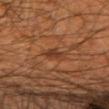biopsy status = imaged on a skin check; not biopsied | anatomic site = the left upper arm | diameter = ≈3 mm | TBP lesion metrics = a classifier nevus-likeness of about 5/100 and lesion-presence confidence of about 100/100 | subject = male, in their mid- to late 40s | imaging modality = ~15 mm tile from a whole-body skin photo | illumination = cross-polarized illumination.